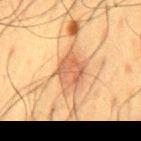Captured during whole-body skin photography for melanoma surveillance; the lesion was not biopsied. The lesion is located on the mid back. Approximately 3.5 mm at its widest. A close-up tile cropped from a whole-body skin photograph, about 15 mm across. The lesion-visualizer software estimated an outline eccentricity of about 0.55 (0 = round, 1 = elongated) and two-axis asymmetry of about 0.2. The software also gave an average lesion color of about L≈48 a*≈21 b*≈32 (CIELAB) and a lesion-to-skin contrast of about 7.5 (normalized; higher = more distinct). And it measured a color-variation rating of about 2.5/10 and radial color variation of about 1. The subject is a male aged 58–62.